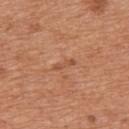notes = total-body-photography surveillance lesion; no biopsy | subject = male, aged around 65 | illumination = white-light | body site = the back | acquisition = 15 mm crop, total-body photography.